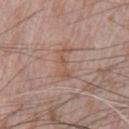Clinical impression:
Part of a total-body skin-imaging series; this lesion was reviewed on a skin check and was not flagged for biopsy.
Image and clinical context:
A lesion tile, about 15 mm wide, cut from a 3D total-body photograph. The patient is a male in their 70s. Longest diameter approximately 4 mm. The lesion is located on the front of the torso.